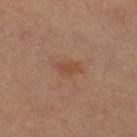The patient is a female in their 70s. The lesion is located on the right thigh. Cropped from a whole-body photographic skin survey; the tile spans about 15 mm.